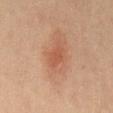{"site": "back", "patient": {"sex": "male", "age_approx": 60}, "image": {"source": "total-body photography crop", "field_of_view_mm": 15}, "lighting": "cross-polarized", "lesion_size": {"long_diameter_mm_approx": 3.0}}